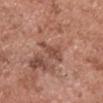<record>
<biopsy_status>not biopsied; imaged during a skin examination</biopsy_status>
<image>
  <source>total-body photography crop</source>
  <field_of_view_mm>15</field_of_view_mm>
</image>
<site>head or neck</site>
<lesion_size>
  <long_diameter_mm_approx>3.0</long_diameter_mm_approx>
</lesion_size>
<patient>
  <sex>male</sex>
  <age_approx>50</age_approx>
</patient>
<automated_metrics>
  <eccentricity>0.75</eccentricity>
  <shape_asymmetry>0.35</shape_asymmetry>
  <nevus_likeness_0_100>0</nevus_likeness_0_100>
  <lesion_detection_confidence_0_100>100</lesion_detection_confidence_0_100>
</automated_metrics>
<lighting>white-light</lighting>
</record>